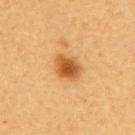Recorded during total-body skin imaging; not selected for excision or biopsy.
The lesion is on the back.
The patient is a male aged 48 to 52.
A 15 mm crop from a total-body photograph taken for skin-cancer surveillance.